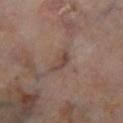Clinical impression: The lesion was photographed on a routine skin check and not biopsied; there is no pathology result. Context: A 15 mm close-up tile from a total-body photography series done for melanoma screening. A male patient, about 70 years old. Located on the leg. This is a cross-polarized tile.